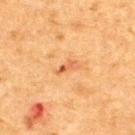Captured during whole-body skin photography for melanoma surveillance; the lesion was not biopsied. A 15 mm close-up tile from a total-body photography series done for melanoma screening. Captured under cross-polarized illumination. The subject is a male aged 48 to 52. From the upper back.